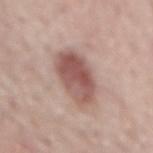The lesion was tiled from a total-body skin photograph and was not biopsied.
A region of skin cropped from a whole-body photographic capture, roughly 15 mm wide.
This is a white-light tile.
Located on the back.
A male patient roughly 70 years of age.
The lesion-visualizer software estimated a shape eccentricity near 0.85. The analysis additionally found a nevus-likeness score of about 95/100.
Measured at roughly 6 mm in maximum diameter.